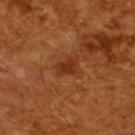diameter=≈2.5 mm
site=the upper back
patient=male, aged 58 to 62
acquisition=~15 mm crop, total-body skin-cancer survey
illumination=cross-polarized illumination